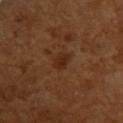Measured at roughly 2.5 mm in maximum diameter.
On the upper back.
Cropped from a total-body skin-imaging series; the visible field is about 15 mm.
A female patient aged around 55.
Captured under cross-polarized illumination.
The lesion-visualizer software estimated an outline eccentricity of about 0.75 (0 = round, 1 = elongated).